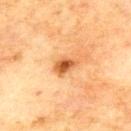follow-up: catalogued during a skin exam; not biopsied.